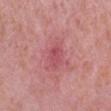Assessment:
The lesion was photographed on a routine skin check and not biopsied; there is no pathology result.
Image and clinical context:
A region of skin cropped from a whole-body photographic capture, roughly 15 mm wide. The lesion is located on the arm. Approximately 2.5 mm at its widest. This is a white-light tile. A female patient approximately 40 years of age.